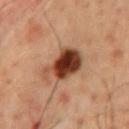- notes: total-body-photography surveillance lesion; no biopsy
- lesion size: about 5.5 mm
- subject: male, approximately 50 years of age
- acquisition: total-body-photography crop, ~15 mm field of view
- anatomic site: the front of the torso